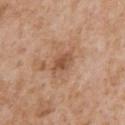Imaged during a routine full-body skin examination; the lesion was not biopsied and no histopathology is available.
Measured at roughly 3 mm in maximum diameter.
On the chest.
Automated tile analysis of the lesion measured an outline eccentricity of about 0.8 (0 = round, 1 = elongated). The software also gave an average lesion color of about L≈51 a*≈22 b*≈32 (CIELAB) and a normalized lesion–skin contrast near 7. It also reported border irregularity of about 2 on a 0–10 scale, a within-lesion color-variation index near 3/10, and radial color variation of about 1.
The subject is a male about 65 years old.
This is a white-light tile.
A 15 mm close-up tile from a total-body photography series done for melanoma screening.